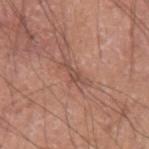biopsy_status: not biopsied; imaged during a skin examination
lesion_size:
  long_diameter_mm_approx: 4.0
site: left upper arm
patient:
  sex: male
  age_approx: 75
image:
  source: total-body photography crop
  field_of_view_mm: 15
automated_metrics:
  border_irregularity_0_10: 5.5
  color_variation_0_10: 0.5
  peripheral_color_asymmetry: 0.0
  nevus_likeness_0_100: 0
  lesion_detection_confidence_0_100: 60